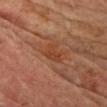Clinical impression:
Captured during whole-body skin photography for melanoma surveillance; the lesion was not biopsied.
Clinical summary:
This image is a 15 mm lesion crop taken from a total-body photograph. Approximately 3 mm at its widest. Captured under cross-polarized illumination. A male patient, approximately 65 years of age. The lesion-visualizer software estimated a shape eccentricity near 0.7 and a symmetry-axis asymmetry near 0.4. The software also gave a lesion color around L≈41 a*≈25 b*≈33 in CIELAB. It also reported a border-irregularity index near 4/10 and a peripheral color-asymmetry measure near 0.5. From the chest.